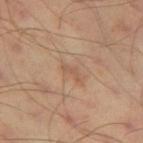No biopsy was performed on this lesion — it was imaged during a full skin examination and was not determined to be concerning.
A roughly 15 mm field-of-view crop from a total-body skin photograph.
A male patient, in their mid- to late 40s.
Located on the left thigh.